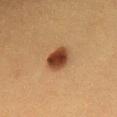follow-up: total-body-photography surveillance lesion; no biopsy
subject: male, aged 38–42
image: ~15 mm tile from a whole-body skin photo
anatomic site: the front of the torso
image-analysis metrics: an area of roughly 7.5 mm², an eccentricity of roughly 0.7, and a shape-asymmetry score of about 0.15 (0 = symmetric); a mean CIELAB color near L≈35 a*≈20 b*≈29, a lesion–skin lightness drop of about 15, and a normalized border contrast of about 12.5